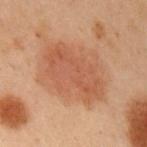* notes — imaged on a skin check; not biopsied
* illumination — cross-polarized
* subject — male, aged 53 to 57
* size — about 7.5 mm
* image source — total-body-photography crop, ~15 mm field of view
* body site — the arm